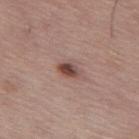biopsy status: imaged on a skin check; not biopsied
subject: female, roughly 60 years of age
lighting: white-light illumination
site: the left thigh
image: 15 mm crop, total-body photography
automated lesion analysis: a lesion area of about 4 mm² and a shape-asymmetry score of about 0.2 (0 = symmetric); an average lesion color of about L≈46 a*≈20 b*≈23 (CIELAB), a lesion–skin lightness drop of about 13, and a normalized border contrast of about 9.5; a nevus-likeness score of about 90/100 and a detector confidence of about 100 out of 100 that the crop contains a lesion
lesion size: ~2.5 mm (longest diameter)